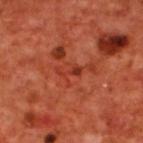illumination: cross-polarized illumination
size: ≈3 mm
body site: the upper back
TBP lesion metrics: a lesion area of about 2.5 mm²; border irregularity of about 7.5 on a 0–10 scale, a color-variation rating of about 0/10, and a peripheral color-asymmetry measure near 0
image source: ~15 mm tile from a whole-body skin photo
patient: male, aged 68 to 72